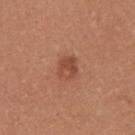The lesion was tiled from a total-body skin photograph and was not biopsied. A female patient, in their mid-20s. Cropped from a total-body skin-imaging series; the visible field is about 15 mm. About 3 mm across. The lesion is on the arm.